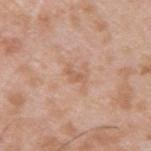workup=no biopsy performed (imaged during a skin exam); image=total-body-photography crop, ~15 mm field of view; subject=male, about 35 years old; illumination=white-light illumination; size=about 2.5 mm; location=the upper back.